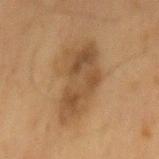This lesion was catalogued during total-body skin photography and was not selected for biopsy. The lesion is on the mid back. Captured under cross-polarized illumination. Automated image analysis of the tile measured a lesion color around L≈40 a*≈15 b*≈30 in CIELAB, roughly 9 lightness units darker than nearby skin, and a normalized lesion–skin contrast near 7.5. It also reported internal color variation of about 4 on a 0–10 scale and radial color variation of about 1.5. A male subject, approximately 60 years of age. Approximately 8 mm at its widest. A 15 mm close-up tile from a total-body photography series done for melanoma screening.